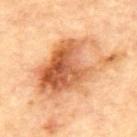This lesion was catalogued during total-body skin photography and was not selected for biopsy. A 15 mm crop from a total-body photograph taken for skin-cancer surveillance. A male patient in their mid-80s or older. Measured at roughly 9 mm in maximum diameter. This is a cross-polarized tile. The total-body-photography lesion software estimated a footprint of about 34 mm² and an outline eccentricity of about 0.75 (0 = round, 1 = elongated). And it measured a lesion–skin lightness drop of about 16 and a normalized border contrast of about 9.5. The analysis additionally found a border-irregularity rating of about 7/10, internal color variation of about 10 on a 0–10 scale, and a peripheral color-asymmetry measure near 4. The analysis additionally found an automated nevus-likeness rating near 50 out of 100 and a lesion-detection confidence of about 100/100. Located on the back.